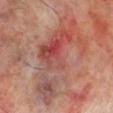workup=no biopsy performed (imaged during a skin exam)
site=the leg
image=total-body-photography crop, ~15 mm field of view
patient=male, roughly 70 years of age
diameter=about 11 mm